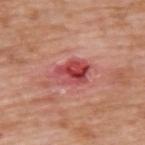follow-up: total-body-photography surveillance lesion; no biopsy
lesion size: about 4 mm
automated lesion analysis: an area of roughly 8.5 mm², an outline eccentricity of about 0.75 (0 = round, 1 = elongated), and two-axis asymmetry of about 0.2; a nevus-likeness score of about 0/100 and a lesion-detection confidence of about 100/100
location: the upper back
subject: male, roughly 60 years of age
image: ~15 mm crop, total-body skin-cancer survey